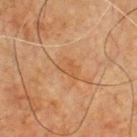The recorded lesion diameter is about 3 mm. A lesion tile, about 15 mm wide, cut from a 3D total-body photograph. The subject is a male aged 63–67. Captured under cross-polarized illumination. The lesion is located on the chest. An algorithmic analysis of the crop reported a mean CIELAB color near L≈48 a*≈20 b*≈35 and about 5 CIELAB-L* units darker than the surrounding skin.